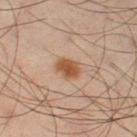The lesion was photographed on a routine skin check and not biopsied; there is no pathology result.
From the left thigh.
Cropped from a total-body skin-imaging series; the visible field is about 15 mm.
Imaged with cross-polarized lighting.
A male subject aged around 40.
Approximately 3 mm at its widest.